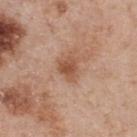Q: Is there a histopathology result?
A: imaged on a skin check; not biopsied
Q: What is the imaging modality?
A: ~15 mm tile from a whole-body skin photo
Q: Who is the patient?
A: male, aged 53–57
Q: What is the anatomic site?
A: the upper back